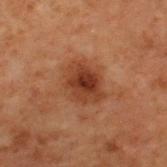Notes:
• follow-up — catalogued during a skin exam; not biopsied
• TBP lesion metrics — an average lesion color of about L≈30 a*≈21 b*≈27 (CIELAB), about 9 CIELAB-L* units darker than the surrounding skin, and a normalized border contrast of about 8.5; a color-variation rating of about 4.5/10 and a peripheral color-asymmetry measure near 1.5
• lesion size — ≈4.5 mm
• image — ~15 mm crop, total-body skin-cancer survey
• subject — male, in their 70s
• location — the upper back
• illumination — cross-polarized